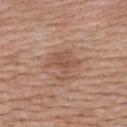Assessment:
Captured during whole-body skin photography for melanoma surveillance; the lesion was not biopsied.
Acquisition and patient details:
Automated tile analysis of the lesion measured a lesion color around L≈53 a*≈21 b*≈28 in CIELAB and a normalized lesion–skin contrast near 5.5. The analysis additionally found a nevus-likeness score of about 10/100 and a lesion-detection confidence of about 100/100. On the upper back. A female subject, about 50 years old. This is a white-light tile. Measured at roughly 3.5 mm in maximum diameter. A 15 mm crop from a total-body photograph taken for skin-cancer surveillance.